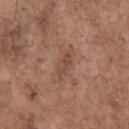notes = no biopsy performed (imaged during a skin exam).